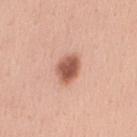  biopsy_status: not biopsied; imaged during a skin examination
  site: arm
  patient:
    sex: female
    age_approx: 30
  image:
    source: total-body photography crop
    field_of_view_mm: 15
  lighting: white-light
  automated_metrics:
    cielab_L: 57
    cielab_a: 24
    cielab_b: 30
    vs_skin_contrast_norm: 10.0
    border_irregularity_0_10: 1.5
    color_variation_0_10: 4.0
    peripheral_color_asymmetry: 1.5
    nevus_likeness_0_100: 100